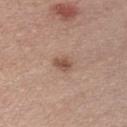A close-up tile cropped from a whole-body skin photograph, about 15 mm across. A male patient, aged 28 to 32.